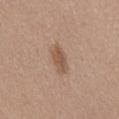This lesion was catalogued during total-body skin photography and was not selected for biopsy. From the abdomen. This is a white-light tile. A male subject aged 48 to 52. A region of skin cropped from a whole-body photographic capture, roughly 15 mm wide. Longest diameter approximately 3.5 mm.